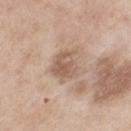biopsy status: imaged on a skin check; not biopsied
patient: female, aged around 55
acquisition: total-body-photography crop, ~15 mm field of view
site: the left thigh